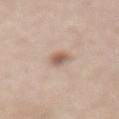Imaged during a routine full-body skin examination; the lesion was not biopsied and no histopathology is available.
A lesion tile, about 15 mm wide, cut from a 3D total-body photograph.
A male subject aged 58–62.
The total-body-photography lesion software estimated border irregularity of about 2.5 on a 0–10 scale and a color-variation rating of about 5/10. The software also gave a nevus-likeness score of about 90/100 and a detector confidence of about 100 out of 100 that the crop contains a lesion.
Measured at roughly 3 mm in maximum diameter.
On the abdomen.
Imaged with white-light lighting.